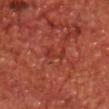Clinical impression: This lesion was catalogued during total-body skin photography and was not selected for biopsy. Background: The tile uses cross-polarized illumination. The lesion-visualizer software estimated a lesion area of about 3.5 mm², a shape eccentricity near 0.85, and a symmetry-axis asymmetry near 0.45. The software also gave a lesion color around L≈36 a*≈33 b*≈31 in CIELAB, a lesion–skin lightness drop of about 5, and a normalized border contrast of about 4.5. It also reported a border-irregularity index near 5.5/10. From the chest. A region of skin cropped from a whole-body photographic capture, roughly 15 mm wide. A male patient aged approximately 70. About 3 mm across.